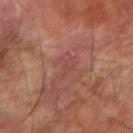No biopsy was performed on this lesion — it was imaged during a full skin examination and was not determined to be concerning. Approximately 3.5 mm at its widest. The tile uses cross-polarized illumination. The total-body-photography lesion software estimated a footprint of about 3.5 mm², a shape eccentricity near 0.9, and two-axis asymmetry of about 0.55. The software also gave border irregularity of about 6 on a 0–10 scale, a color-variation rating of about 1/10, and radial color variation of about 0. From the right forearm. A male subject aged around 65. A 15 mm close-up tile from a total-body photography series done for melanoma screening.